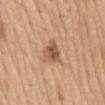Q: Was a biopsy performed?
A: catalogued during a skin exam; not biopsied
Q: Automated lesion metrics?
A: a border-irregularity index near 3/10, a color-variation rating of about 5/10, and radial color variation of about 1.5; an automated nevus-likeness rating near 65 out of 100
Q: How large is the lesion?
A: ~3 mm (longest diameter)
Q: How was the tile lit?
A: white-light illumination
Q: What is the imaging modality?
A: 15 mm crop, total-body photography
Q: What are the patient's age and sex?
A: female, about 65 years old
Q: What is the anatomic site?
A: the back